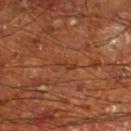Part of a total-body skin-imaging series; this lesion was reviewed on a skin check and was not flagged for biopsy. A male subject aged 63–67. Located on the left lower leg. Cropped from a total-body skin-imaging series; the visible field is about 15 mm. About 3 mm across. Captured under cross-polarized illumination. The total-body-photography lesion software estimated a shape eccentricity near 0.95. The analysis additionally found an average lesion color of about L≈36 a*≈23 b*≈31 (CIELAB) and a normalized border contrast of about 5. And it measured an automated nevus-likeness rating near 0 out of 100 and a detector confidence of about 95 out of 100 that the crop contains a lesion.